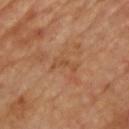workup — total-body-photography surveillance lesion; no biopsy | imaging modality — ~15 mm tile from a whole-body skin photo | patient — male, roughly 60 years of age | site — the chest | diameter — about 3 mm.